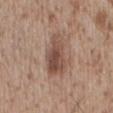The lesion was tiled from a total-body skin photograph and was not biopsied.